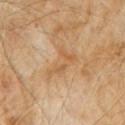The lesion was photographed on a routine skin check and not biopsied; there is no pathology result. The lesion is on the abdomen. The subject is a male aged 58–62. The tile uses cross-polarized illumination. The lesion's longest dimension is about 4 mm. A roughly 15 mm field-of-view crop from a total-body skin photograph.